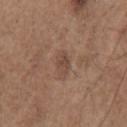Recorded during total-body skin imaging; not selected for excision or biopsy.
Imaged with white-light lighting.
On the chest.
Longest diameter approximately 2.5 mm.
A male patient aged 68–72.
A 15 mm crop from a total-body photograph taken for skin-cancer surveillance.